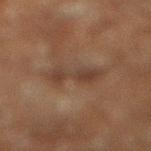Q: Is there a histopathology result?
A: catalogued during a skin exam; not biopsied
Q: What lighting was used for the tile?
A: cross-polarized
Q: Where on the body is the lesion?
A: the right lower leg
Q: Who is the patient?
A: male, aged approximately 60
Q: How was this image acquired?
A: ~15 mm tile from a whole-body skin photo